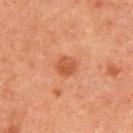biopsy status: imaged on a skin check; not biopsied